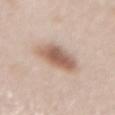image: ~15 mm tile from a whole-body skin photo
body site: the front of the torso
patient: male, in their 60s